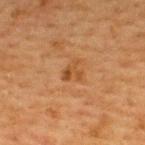<lesion>
  <biopsy_status>not biopsied; imaged during a skin examination</biopsy_status>
  <site>upper back</site>
  <automated_metrics>
    <area_mm2_approx>3.0</area_mm2_approx>
    <shape_asymmetry>0.45</shape_asymmetry>
    <border_irregularity_0_10>5.5</border_irregularity_0_10>
    <peripheral_color_asymmetry>0.0</peripheral_color_asymmetry>
  </automated_metrics>
  <image>
    <source>total-body photography crop</source>
    <field_of_view_mm>15</field_of_view_mm>
  </image>
  <patient>
    <sex>female</sex>
    <age_approx>40</age_approx>
  </patient>
  <lesion_size>
    <long_diameter_mm_approx>2.5</long_diameter_mm_approx>
  </lesion_size>
  <lighting>cross-polarized</lighting>
</lesion>